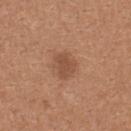The lesion was tiled from a total-body skin photograph and was not biopsied.
Imaged with white-light lighting.
The lesion is on the upper back.
A 15 mm crop from a total-body photograph taken for skin-cancer surveillance.
The total-body-photography lesion software estimated an area of roughly 5.5 mm², an outline eccentricity of about 0.6 (0 = round, 1 = elongated), and a shape-asymmetry score of about 0.25 (0 = symmetric). The software also gave a classifier nevus-likeness of about 30/100.
Longest diameter approximately 3 mm.
A female subject aged 38–42.